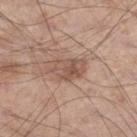Notes:
• biopsy status: imaged on a skin check; not biopsied
• size: ≈4 mm
• body site: the right lower leg
• subject: male, approximately 55 years of age
• illumination: white-light
• acquisition: total-body-photography crop, ~15 mm field of view
• automated lesion analysis: a lesion area of about 8 mm² and an eccentricity of roughly 0.8; an automated nevus-likeness rating near 5 out of 100 and lesion-presence confidence of about 100/100